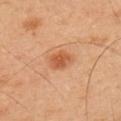Context:
A male patient, roughly 45 years of age. This image is a 15 mm lesion crop taken from a total-body photograph. From the left upper arm. The tile uses cross-polarized illumination.